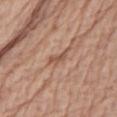{"biopsy_status": "not biopsied; imaged during a skin examination", "site": "chest", "image": {"source": "total-body photography crop", "field_of_view_mm": 15}, "patient": {"sex": "male", "age_approx": 70}}